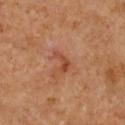Imaged during a routine full-body skin examination; the lesion was not biopsied and no histopathology is available.
Imaged with cross-polarized lighting.
The lesion is located on the chest.
The patient is a female aged approximately 50.
A roughly 15 mm field-of-view crop from a total-body skin photograph.
Approximately 3 mm at its widest.